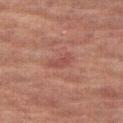{
  "biopsy_status": "not biopsied; imaged during a skin examination",
  "automated_metrics": {
    "border_irregularity_0_10": 4.0,
    "color_variation_0_10": 0.5,
    "nevus_likeness_0_100": 0,
    "lesion_detection_confidence_0_100": 100
  },
  "site": "right thigh",
  "lighting": "cross-polarized",
  "image": {
    "source": "total-body photography crop",
    "field_of_view_mm": 15
  },
  "patient": {
    "sex": "female",
    "age_approx": 70
  }
}